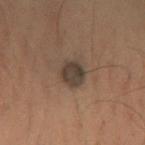Clinical summary: A male patient, in their mid-50s. Cropped from a total-body skin-imaging series; the visible field is about 15 mm. The tile uses cross-polarized illumination. Longest diameter approximately 3 mm. Located on the right forearm.